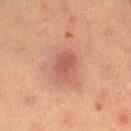workup: imaged on a skin check; not biopsied
location: the right thigh
patient: female, aged approximately 55
automated lesion analysis: a shape eccentricity near 0.7 and a shape-asymmetry score of about 0.2 (0 = symmetric); a mean CIELAB color near L≈44 a*≈22 b*≈23, a lesion–skin lightness drop of about 7, and a lesion-to-skin contrast of about 6 (normalized; higher = more distinct)
acquisition: total-body-photography crop, ~15 mm field of view